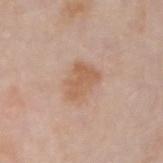Impression: Part of a total-body skin-imaging series; this lesion was reviewed on a skin check and was not flagged for biopsy. Context: The patient is a male roughly 75 years of age. A 15 mm close-up tile from a total-body photography series done for melanoma screening. Automated tile analysis of the lesion measured a lesion area of about 9 mm², an outline eccentricity of about 0.75 (0 = round, 1 = elongated), and a symmetry-axis asymmetry near 0.25. The software also gave an average lesion color of about L≈59 a*≈19 b*≈32 (CIELAB) and a normalized border contrast of about 6.5. From the right forearm.